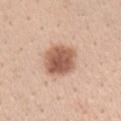follow-up: no biopsy performed (imaged during a skin exam) | acquisition: ~15 mm tile from a whole-body skin photo | lighting: white-light | patient: female, roughly 30 years of age | location: the chest.